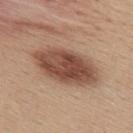A female patient, aged 38–42. A 15 mm close-up tile from a total-body photography series done for melanoma screening. The total-body-photography lesion software estimated an area of roughly 24 mm² and an eccentricity of roughly 0.85. It also reported a lesion color around L≈49 a*≈21 b*≈28 in CIELAB, a lesion–skin lightness drop of about 15, and a lesion-to-skin contrast of about 10 (normalized; higher = more distinct). The analysis additionally found a classifier nevus-likeness of about 95/100 and a lesion-detection confidence of about 100/100. On the back. Measured at roughly 8 mm in maximum diameter.